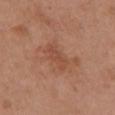biopsy status: no biopsy performed (imaged during a skin exam) | anatomic site: the chest | illumination: white-light | subject: female, roughly 65 years of age | acquisition: ~15 mm crop, total-body skin-cancer survey.